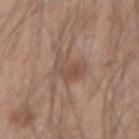Notes:
- biopsy status: imaged on a skin check; not biopsied
- location: the right forearm
- acquisition: ~15 mm tile from a whole-body skin photo
- patient: male, about 45 years old
- size: about 4 mm
- illumination: white-light